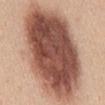Clinical impression: Recorded during total-body skin imaging; not selected for excision or biopsy. Image and clinical context: Captured under white-light illumination. On the back. A female patient, aged 43–47. A 15 mm crop from a total-body photograph taken for skin-cancer surveillance. Measured at roughly 16 mm in maximum diameter.